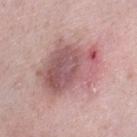- workup · total-body-photography surveillance lesion; no biopsy
- image-analysis metrics · an average lesion color of about L≈56 a*≈23 b*≈19 (CIELAB) and roughly 12 lightness units darker than nearby skin; a border-irregularity rating of about 3.5/10 and peripheral color asymmetry of about 2.5; a lesion-detection confidence of about 100/100
- site · the left lower leg
- patient · female, aged around 40
- tile lighting · white-light
- acquisition · 15 mm crop, total-body photography